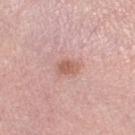This lesion was catalogued during total-body skin photography and was not selected for biopsy.
A roughly 15 mm field-of-view crop from a total-body skin photograph.
Measured at roughly 2.5 mm in maximum diameter.
The lesion is located on the right thigh.
A female patient, in their mid-60s.